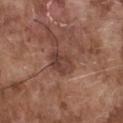<lesion>
  <biopsy_status>not biopsied; imaged during a skin examination</biopsy_status>
  <patient>
    <sex>male</sex>
    <age_approx>75</age_approx>
  </patient>
  <lighting>white-light</lighting>
  <site>chest</site>
  <image>
    <source>total-body photography crop</source>
    <field_of_view_mm>15</field_of_view_mm>
  </image>
  <lesion_size>
    <long_diameter_mm_approx>3.5</long_diameter_mm_approx>
  </lesion_size>
</lesion>